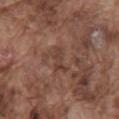{"biopsy_status": "not biopsied; imaged during a skin examination", "lesion_size": {"long_diameter_mm_approx": 3.0}, "image": {"source": "total-body photography crop", "field_of_view_mm": 15}, "patient": {"sex": "male", "age_approx": 75}, "site": "mid back", "lighting": "white-light", "automated_metrics": {"border_irregularity_0_10": 7.0, "color_variation_0_10": 0.0, "peripheral_color_asymmetry": 0.0}}